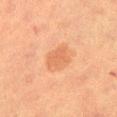Clinical impression: The lesion was photographed on a routine skin check and not biopsied; there is no pathology result. Clinical summary: This is a cross-polarized tile. The lesion-visualizer software estimated a footprint of about 6.5 mm² and a shape eccentricity near 0.45. The analysis additionally found a mean CIELAB color near L≈52 a*≈22 b*≈33 and roughly 7 lightness units darker than nearby skin. The software also gave a detector confidence of about 100 out of 100 that the crop contains a lesion. The lesion's longest dimension is about 3 mm. Cropped from a total-body skin-imaging series; the visible field is about 15 mm. A female patient aged 53–57. The lesion is on the left thigh.